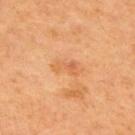{"biopsy_status": "not biopsied; imaged during a skin examination", "patient": {"sex": "male", "age_approx": 65}, "lesion_size": {"long_diameter_mm_approx": 3.5}, "image": {"source": "total-body photography crop", "field_of_view_mm": 15}, "lighting": "cross-polarized", "site": "upper back"}